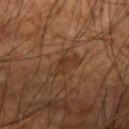<tbp_lesion>
<biopsy_status>not biopsied; imaged during a skin examination</biopsy_status>
<site>left upper arm</site>
<patient>
  <sex>male</sex>
  <age_approx>60</age_approx>
</patient>
<image>
  <source>total-body photography crop</source>
  <field_of_view_mm>15</field_of_view_mm>
</image>
<lesion_size>
  <long_diameter_mm_approx>3.0</long_diameter_mm_approx>
</lesion_size>
</tbp_lesion>